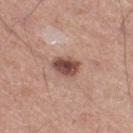Part of a total-body skin-imaging series; this lesion was reviewed on a skin check and was not flagged for biopsy. The lesion is on the right thigh. A close-up tile cropped from a whole-body skin photograph, about 15 mm across. A male patient about 50 years old.